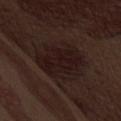<record>
<biopsy_status>not biopsied; imaged during a skin examination</biopsy_status>
<image>
  <source>total-body photography crop</source>
  <field_of_view_mm>15</field_of_view_mm>
</image>
<patient>
  <sex>male</sex>
  <age_approx>70</age_approx>
</patient>
<lighting>white-light</lighting>
<automated_metrics>
  <area_mm2_approx>24.0</area_mm2_approx>
  <eccentricity>0.7</eccentricity>
  <shape_asymmetry>0.15</shape_asymmetry>
  <cielab_L>16</cielab_L>
  <cielab_a>14</cielab_a>
  <cielab_b>14</cielab_b>
  <vs_skin_darker_L>5.0</vs_skin_darker_L>
  <vs_skin_contrast_norm>8.5</vs_skin_contrast_norm>
</automated_metrics>
<site>abdomen</site>
<lesion_size>
  <long_diameter_mm_approx>7.0</long_diameter_mm_approx>
</lesion_size>
</record>